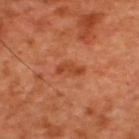Clinical impression:
Recorded during total-body skin imaging; not selected for excision or biopsy.
Background:
Cropped from a total-body skin-imaging series; the visible field is about 15 mm. From the upper back. The tile uses cross-polarized illumination. The subject is a male roughly 50 years of age.